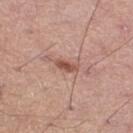The tile uses white-light illumination. A lesion tile, about 15 mm wide, cut from a 3D total-body photograph. The lesion is on the right thigh. A male subject, aged approximately 50. Approximately 3 mm at its widest. The total-body-photography lesion software estimated a mean CIELAB color near L≈52 a*≈23 b*≈27, roughly 12 lightness units darker than nearby skin, and a normalized lesion–skin contrast near 8. The analysis additionally found a classifier nevus-likeness of about 85/100 and a detector confidence of about 100 out of 100 that the crop contains a lesion.